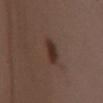Part of a total-body skin-imaging series; this lesion was reviewed on a skin check and was not flagged for biopsy.
The tile uses white-light illumination.
Cropped from a total-body skin-imaging series; the visible field is about 15 mm.
A female patient, in their mid-30s.
The lesion-visualizer software estimated roughly 10 lightness units darker than nearby skin and a normalized lesion–skin contrast near 9.5. And it measured internal color variation of about 2.5 on a 0–10 scale and radial color variation of about 1. It also reported a classifier nevus-likeness of about 100/100 and a lesion-detection confidence of about 100/100.
About 4 mm across.
From the front of the torso.